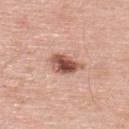This is a white-light tile. A male subject, roughly 60 years of age. The lesion's longest dimension is about 4 mm. Located on the upper back. Cropped from a total-body skin-imaging series; the visible field is about 15 mm.